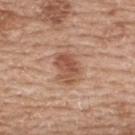notes: total-body-photography surveillance lesion; no biopsy
tile lighting: white-light
body site: the upper back
image: ~15 mm tile from a whole-body skin photo
image-analysis metrics: a mean CIELAB color near L≈53 a*≈22 b*≈31; an automated nevus-likeness rating near 80 out of 100 and a detector confidence of about 100 out of 100 that the crop contains a lesion
subject: female, aged 58–62